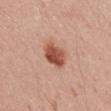Assessment:
No biopsy was performed on this lesion — it was imaged during a full skin examination and was not determined to be concerning.
Context:
Cropped from a total-body skin-imaging series; the visible field is about 15 mm. Located on the back. The subject is a male aged around 30.